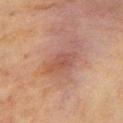Recorded during total-body skin imaging; not selected for excision or biopsy. A region of skin cropped from a whole-body photographic capture, roughly 15 mm wide. This is a cross-polarized tile. The subject is a female in their mid-50s. From the chest.